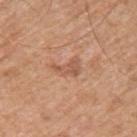Imaged during a routine full-body skin examination; the lesion was not biopsied and no histopathology is available. Imaged with white-light lighting. Cropped from a total-body skin-imaging series; the visible field is about 15 mm. The lesion-visualizer software estimated a footprint of about 4.5 mm², an eccentricity of roughly 0.8, and a symmetry-axis asymmetry near 0.5. And it measured a within-lesion color-variation index near 2/10. A male patient aged approximately 65. Approximately 3 mm at its widest. Located on the left upper arm.